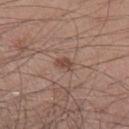Part of a total-body skin-imaging series; this lesion was reviewed on a skin check and was not flagged for biopsy.
The lesion is on the left lower leg.
About 2.5 mm across.
A 15 mm close-up extracted from a 3D total-body photography capture.
Imaged with white-light lighting.
A male patient, about 30 years old.